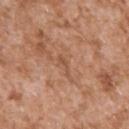<case>
  <biopsy_status>not biopsied; imaged during a skin examination</biopsy_status>
  <automated_metrics>
    <nevus_likeness_0_100>0</nevus_likeness_0_100>
  </automated_metrics>
  <lighting>white-light</lighting>
  <image>
    <source>total-body photography crop</source>
    <field_of_view_mm>15</field_of_view_mm>
  </image>
  <patient>
    <sex>male</sex>
    <age_approx>45</age_approx>
  </patient>
  <lesion_size>
    <long_diameter_mm_approx>3.0</long_diameter_mm_approx>
  </lesion_size>
  <site>left upper arm</site>
</case>